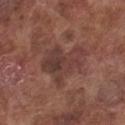Captured during whole-body skin photography for melanoma surveillance; the lesion was not biopsied.
The lesion's longest dimension is about 5.5 mm.
Located on the chest.
A male patient, roughly 75 years of age.
This image is a 15 mm lesion crop taken from a total-body photograph.